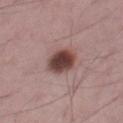Captured under white-light illumination.
From the right thigh.
The total-body-photography lesion software estimated a color-variation rating of about 5/10 and radial color variation of about 1.5. And it measured a nevus-likeness score of about 90/100.
This image is a 15 mm lesion crop taken from a total-body photograph.
A male subject aged around 50.
Longest diameter approximately 3.5 mm.